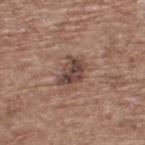<lesion>
  <lesion_size>
    <long_diameter_mm_approx>3.5</long_diameter_mm_approx>
  </lesion_size>
  <lighting>white-light</lighting>
  <patient>
    <sex>male</sex>
    <age_approx>70</age_approx>
  </patient>
  <image>
    <source>total-body photography crop</source>
    <field_of_view_mm>15</field_of_view_mm>
  </image>
  <site>upper back</site>
  <automated_metrics>
    <area_mm2_approx>7.0</area_mm2_approx>
    <eccentricity>0.7</eccentricity>
    <cielab_L>43</cielab_L>
    <cielab_a>18</cielab_a>
    <cielab_b>22</cielab_b>
    <nevus_likeness_0_100>35</nevus_likeness_0_100>
    <lesion_detection_confidence_0_100>100</lesion_detection_confidence_0_100>
  </automated_metrics>
</lesion>